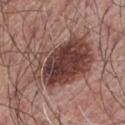Q: Was a biopsy performed?
A: catalogued during a skin exam; not biopsied
Q: How was this image acquired?
A: total-body-photography crop, ~15 mm field of view
Q: What is the lesion's diameter?
A: ≈8.5 mm
Q: Patient demographics?
A: male, aged around 65
Q: Where on the body is the lesion?
A: the front of the torso
Q: What did automated image analysis measure?
A: an average lesion color of about L≈41 a*≈20 b*≈22 (CIELAB) and a normalized border contrast of about 10.5; border irregularity of about 5 on a 0–10 scale and a peripheral color-asymmetry measure near 3